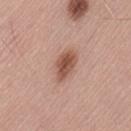Q: Was a biopsy performed?
A: catalogued during a skin exam; not biopsied
Q: Automated lesion metrics?
A: a lesion color around L≈53 a*≈23 b*≈28 in CIELAB, about 13 CIELAB-L* units darker than the surrounding skin, and a lesion-to-skin contrast of about 9 (normalized; higher = more distinct)
Q: Where on the body is the lesion?
A: the back
Q: Lesion size?
A: ~4 mm (longest diameter)
Q: How was the tile lit?
A: white-light
Q: Who is the patient?
A: male, roughly 55 years of age
Q: How was this image acquired?
A: ~15 mm tile from a whole-body skin photo